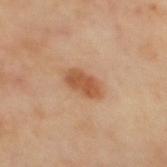Clinical impression: Recorded during total-body skin imaging; not selected for excision or biopsy. Acquisition and patient details: On the mid back. A region of skin cropped from a whole-body photographic capture, roughly 15 mm wide. Longest diameter approximately 4 mm.